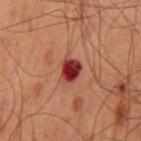location: the mid back
lighting: cross-polarized illumination
TBP lesion metrics: a footprint of about 5.5 mm², an outline eccentricity of about 0.6 (0 = round, 1 = elongated), and two-axis asymmetry of about 0.2; a lesion–skin lightness drop of about 14; border irregularity of about 2 on a 0–10 scale, a color-variation rating of about 5.5/10, and peripheral color asymmetry of about 1.5; a nevus-likeness score of about 0/100 and lesion-presence confidence of about 100/100
imaging modality: total-body-photography crop, ~15 mm field of view
size: ~3 mm (longest diameter)
patient: male, aged approximately 60